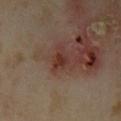biopsy status=no biopsy performed (imaged during a skin exam)